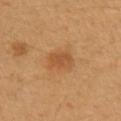Impression: Captured during whole-body skin photography for melanoma surveillance; the lesion was not biopsied. Clinical summary: The recorded lesion diameter is about 3 mm. A close-up tile cropped from a whole-body skin photograph, about 15 mm across. Automated tile analysis of the lesion measured roughly 8 lightness units darker than nearby skin and a normalized border contrast of about 6. A female patient roughly 40 years of age. The tile uses cross-polarized illumination. The lesion is on the arm.